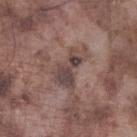Findings:
– follow-up · imaged on a skin check; not biopsied
– body site · the left lower leg
– image-analysis metrics · an automated nevus-likeness rating near 0 out of 100 and a lesion-detection confidence of about 90/100
– subject · male, aged 73 to 77
– imaging modality · 15 mm crop, total-body photography
– size · ≈5 mm